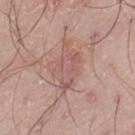| feature | finding |
|---|---|
| biopsy status | total-body-photography surveillance lesion; no biopsy |
| lighting | white-light illumination |
| patient | male, approximately 20 years of age |
| automated metrics | roughly 8 lightness units darker than nearby skin and a lesion-to-skin contrast of about 5.5 (normalized; higher = more distinct); border irregularity of about 4.5 on a 0–10 scale, internal color variation of about 3 on a 0–10 scale, and a peripheral color-asymmetry measure near 1 |
| diameter | ~5.5 mm (longest diameter) |
| image | ~15 mm crop, total-body skin-cancer survey |
| body site | the left thigh |